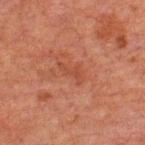automated metrics — a lesion area of about 3.5 mm² and a shape eccentricity near 0.9; image — ~15 mm crop, total-body skin-cancer survey; tile lighting — cross-polarized; size — ≈3.5 mm; patient — male, aged 58–62; location — the upper back.